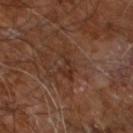Q: Was this lesion biopsied?
A: no biopsy performed (imaged during a skin exam)
Q: Automated lesion metrics?
A: a lesion area of about 4.5 mm² and a shape-asymmetry score of about 0.4 (0 = symmetric); a normalized border contrast of about 6.5; an automated nevus-likeness rating near 0 out of 100 and a detector confidence of about 90 out of 100 that the crop contains a lesion
Q: How large is the lesion?
A: ≈3.5 mm
Q: How was the tile lit?
A: cross-polarized
Q: What are the patient's age and sex?
A: male, aged 58 to 62
Q: What kind of image is this?
A: ~15 mm tile from a whole-body skin photo
Q: What is the anatomic site?
A: the leg